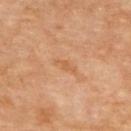A close-up tile cropped from a whole-body skin photograph, about 15 mm across.
Located on the back.
A male subject, aged around 70.